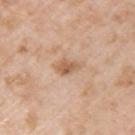Impression:
This lesion was catalogued during total-body skin photography and was not selected for biopsy.
Clinical summary:
The lesion is on the left upper arm. About 2.5 mm across. Captured under white-light illumination. A region of skin cropped from a whole-body photographic capture, roughly 15 mm wide. An algorithmic analysis of the crop reported a border-irregularity rating of about 2.5/10, a color-variation rating of about 2.5/10, and peripheral color asymmetry of about 1. The software also gave a nevus-likeness score of about 5/100. A male subject, about 60 years old.